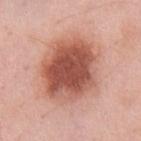The lesion was tiled from a total-body skin photograph and was not biopsied. This image is a 15 mm lesion crop taken from a total-body photograph. A male subject approximately 60 years of age. About 7.5 mm across. The lesion is located on the chest. This is a white-light tile.